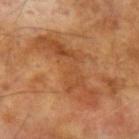follow-up — catalogued during a skin exam; not biopsied | TBP lesion metrics — a mean CIELAB color near L≈48 a*≈25 b*≈38, a lesion–skin lightness drop of about 8, and a normalized border contrast of about 6.5; border irregularity of about 9 on a 0–10 scale, a color-variation rating of about 4.5/10, and radial color variation of about 1.5; lesion-presence confidence of about 85/100 | patient — male, aged approximately 70 | image source — 15 mm crop, total-body photography | anatomic site — the left upper arm | tile lighting — cross-polarized | lesion diameter — ≈10 mm.